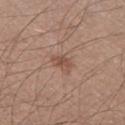site: the left thigh | image: 15 mm crop, total-body photography | patient: male, aged around 45 | automated lesion analysis: a footprint of about 3.5 mm² and a shape-asymmetry score of about 0.3 (0 = symmetric); a border-irregularity index near 2.5/10 and a peripheral color-asymmetry measure near 1; an automated nevus-likeness rating near 0 out of 100 and a detector confidence of about 100 out of 100 that the crop contains a lesion | lighting: white-light illumination.